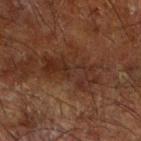Clinical impression: The lesion was tiled from a total-body skin photograph and was not biopsied. Image and clinical context: Cropped from a total-body skin-imaging series; the visible field is about 15 mm. A male patient, in their mid- to late 60s. The lesion's longest dimension is about 7 mm. On the right forearm.